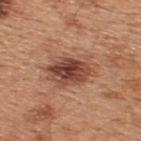Assessment:
The lesion was photographed on a routine skin check and not biopsied; there is no pathology result.
Context:
Cropped from a total-body skin-imaging series; the visible field is about 15 mm. Located on the upper back. A male subject, aged around 45. Captured under white-light illumination. An algorithmic analysis of the crop reported a footprint of about 16 mm², an eccentricity of roughly 0.75, and a shape-asymmetry score of about 0.2 (0 = symmetric). And it measured a lesion color around L≈47 a*≈24 b*≈29 in CIELAB, roughly 13 lightness units darker than nearby skin, and a normalized border contrast of about 9.5. The analysis additionally found a classifier nevus-likeness of about 85/100. Longest diameter approximately 6 mm.